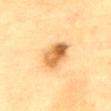Notes:
• subject · female, aged around 80
• size · ≈4.5 mm
• imaging modality · ~15 mm crop, total-body skin-cancer survey
• automated lesion analysis · a border-irregularity index near 2/10, a color-variation rating of about 8.5/10, and radial color variation of about 3.5; a detector confidence of about 100 out of 100 that the crop contains a lesion
• tile lighting · cross-polarized illumination
• location · the mid back